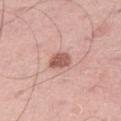Assessment: This lesion was catalogued during total-body skin photography and was not selected for biopsy. Image and clinical context: Cropped from a total-body skin-imaging series; the visible field is about 15 mm. The total-body-photography lesion software estimated an area of roughly 5 mm², an outline eccentricity of about 0.65 (0 = round, 1 = elongated), and a symmetry-axis asymmetry near 0.15. It also reported a border-irregularity index near 1.5/10, a color-variation rating of about 3/10, and a peripheral color-asymmetry measure near 1. The analysis additionally found a lesion-detection confidence of about 100/100. The subject is a male aged around 45. The lesion is on the leg. Approximately 2.5 mm at its widest.